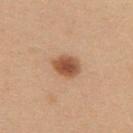This lesion was catalogued during total-body skin photography and was not selected for biopsy. A 15 mm crop from a total-body photograph taken for skin-cancer surveillance. The lesion's longest dimension is about 3.5 mm. An algorithmic analysis of the crop reported a lesion area of about 7 mm² and a shape eccentricity near 0.6. The software also gave a lesion color around L≈53 a*≈23 b*≈34 in CIELAB, roughly 14 lightness units darker than nearby skin, and a normalized border contrast of about 9.5. And it measured border irregularity of about 1.5 on a 0–10 scale and peripheral color asymmetry of about 1. The lesion is located on the upper back. A female subject aged 23–27.